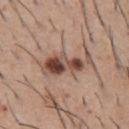Clinical impression: This lesion was catalogued during total-body skin photography and was not selected for biopsy. Background: The lesion is located on the chest. A 15 mm close-up extracted from a 3D total-body photography capture. The lesion's longest dimension is about 6.5 mm. A male subject aged 58 to 62.